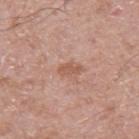Assessment: Imaged during a routine full-body skin examination; the lesion was not biopsied and no histopathology is available. Background: Automated image analysis of the tile measured border irregularity of about 3 on a 0–10 scale. Imaged with white-light lighting. A close-up tile cropped from a whole-body skin photograph, about 15 mm across. A male patient about 50 years old. The lesion is on the left upper arm.